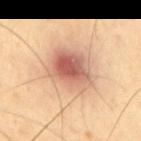Recorded during total-body skin imaging; not selected for excision or biopsy. On the mid back. A 15 mm close-up extracted from a 3D total-body photography capture. The patient is a male aged 38–42. Automated image analysis of the tile measured an average lesion color of about L≈68 a*≈20 b*≈32 (CIELAB), about 11 CIELAB-L* units darker than the surrounding skin, and a lesion-to-skin contrast of about 7 (normalized; higher = more distinct). It also reported a classifier nevus-likeness of about 10/100 and a lesion-detection confidence of about 100/100. This is a cross-polarized tile.